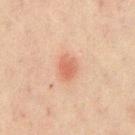Recorded during total-body skin imaging; not selected for excision or biopsy.
The lesion is located on the mid back.
The lesion's longest dimension is about 3 mm.
A roughly 15 mm field-of-view crop from a total-body skin photograph.
The patient is a male aged 68–72.
Captured under cross-polarized illumination.